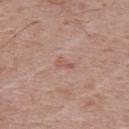Assessment:
Imaged during a routine full-body skin examination; the lesion was not biopsied and no histopathology is available.
Background:
This image is a 15 mm lesion crop taken from a total-body photograph. A male patient approximately 70 years of age. Located on the back.